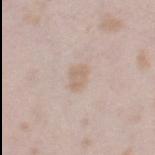follow-up=catalogued during a skin exam; not biopsied | location=the right thigh | subject=female, aged 23–27 | acquisition=~15 mm crop, total-body skin-cancer survey | tile lighting=white-light | size=about 2.5 mm | automated lesion analysis=a lesion area of about 3.5 mm² and two-axis asymmetry of about 0.35; an average lesion color of about L≈64 a*≈14 b*≈26 (CIELAB), about 7 CIELAB-L* units darker than the surrounding skin, and a normalized lesion–skin contrast near 5.5; a border-irregularity rating of about 3/10, a color-variation rating of about 1/10, and radial color variation of about 0.5; an automated nevus-likeness rating near 5 out of 100 and lesion-presence confidence of about 100/100.